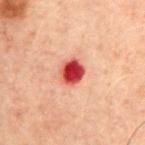Q: Was a biopsy performed?
A: no biopsy performed (imaged during a skin exam)
Q: What is the anatomic site?
A: the chest
Q: Patient demographics?
A: male, about 60 years old
Q: What is the lesion's diameter?
A: ≈3 mm
Q: How was this image acquired?
A: ~15 mm crop, total-body skin-cancer survey
Q: Illumination type?
A: cross-polarized
Q: What did automated image analysis measure?
A: an average lesion color of about L≈38 a*≈35 b*≈26 (CIELAB) and roughly 18 lightness units darker than nearby skin; border irregularity of about 1 on a 0–10 scale, internal color variation of about 4 on a 0–10 scale, and radial color variation of about 1; an automated nevus-likeness rating near 0 out of 100